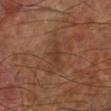| key | value |
|---|---|
| biopsy status | catalogued during a skin exam; not biopsied |
| diameter | about 3.5 mm |
| site | the left lower leg |
| patient | male, in their 60s |
| image source | ~15 mm tile from a whole-body skin photo |
| TBP lesion metrics | a lesion color around L≈36 a*≈20 b*≈28 in CIELAB, about 6 CIELAB-L* units darker than the surrounding skin, and a normalized border contrast of about 5.5; border irregularity of about 4 on a 0–10 scale, a color-variation rating of about 1.5/10, and a peripheral color-asymmetry measure near 0.5 |
| tile lighting | cross-polarized |